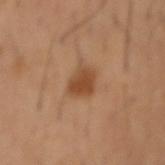Clinical impression: Imaged during a routine full-body skin examination; the lesion was not biopsied and no histopathology is available. Acquisition and patient details: This is a cross-polarized tile. An algorithmic analysis of the crop reported a lesion area of about 6.5 mm², a shape eccentricity near 0.55, and two-axis asymmetry of about 0.25. It also reported radial color variation of about 1. And it measured a classifier nevus-likeness of about 95/100. On the right forearm. A lesion tile, about 15 mm wide, cut from a 3D total-body photograph. A male subject, aged 48 to 52. Approximately 3 mm at its widest.